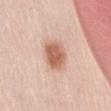Clinical impression:
The lesion was tiled from a total-body skin photograph and was not biopsied.
Acquisition and patient details:
About 4 mm across. A male patient aged approximately 50. The tile uses white-light illumination. The lesion-visualizer software estimated a footprint of about 8.5 mm², a shape eccentricity near 0.65, and two-axis asymmetry of about 0.2. It also reported a mean CIELAB color near L≈61 a*≈23 b*≈31, a lesion–skin lightness drop of about 14, and a lesion-to-skin contrast of about 9 (normalized; higher = more distinct). The software also gave a border-irregularity rating of about 1.5/10, a color-variation rating of about 2.5/10, and radial color variation of about 1. From the abdomen. A roughly 15 mm field-of-view crop from a total-body skin photograph.